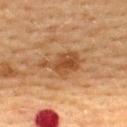Recorded during total-body skin imaging; not selected for excision or biopsy.
The tile uses cross-polarized illumination.
Located on the upper back.
A lesion tile, about 15 mm wide, cut from a 3D total-body photograph.
The patient is a female about 55 years old.